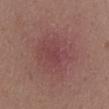The lesion was tiled from a total-body skin photograph and was not biopsied. A 15 mm close-up extracted from a 3D total-body photography capture. The tile uses white-light illumination. A male patient aged 53–57. Approximately 7 mm at its widest. Located on the chest.